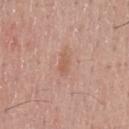<lesion>
  <biopsy_status>not biopsied; imaged during a skin examination</biopsy_status>
  <automated_metrics>
    <area_mm2_approx>3.0</area_mm2_approx>
    <shape_asymmetry>0.35</shape_asymmetry>
    <cielab_L>58</cielab_L>
    <cielab_a>22</cielab_a>
    <cielab_b>29</cielab_b>
    <vs_skin_darker_L>7.0</vs_skin_darker_L>
    <peripheral_color_asymmetry>0.0</peripheral_color_asymmetry>
    <nevus_likeness_0_100>0</nevus_likeness_0_100>
  </automated_metrics>
  <lesion_size>
    <long_diameter_mm_approx>2.5</long_diameter_mm_approx>
  </lesion_size>
  <site>chest</site>
  <image>
    <source>total-body photography crop</source>
    <field_of_view_mm>15</field_of_view_mm>
  </image>
  <lighting>white-light</lighting>
  <patient>
    <sex>male</sex>
    <age_approx>45</age_approx>
  </patient>
</lesion>